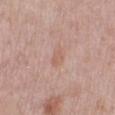The lesion was photographed on a routine skin check and not biopsied; there is no pathology result. A close-up tile cropped from a whole-body skin photograph, about 15 mm across. A female subject, aged 38 to 42. The lesion is located on the leg.